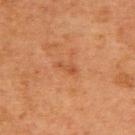biopsy status = imaged on a skin check; not biopsied
patient = female, about 50 years old
lesion diameter = about 2.5 mm
location = the upper back
acquisition = ~15 mm tile from a whole-body skin photo
automated lesion analysis = a mean CIELAB color near L≈44 a*≈24 b*≈34 and a normalized lesion–skin contrast near 5.5; a lesion-detection confidence of about 100/100
tile lighting = cross-polarized illumination